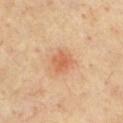Assessment:
The lesion was tiled from a total-body skin photograph and was not biopsied.